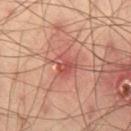Assessment:
Part of a total-body skin-imaging series; this lesion was reviewed on a skin check and was not flagged for biopsy.
Background:
A 15 mm close-up tile from a total-body photography series done for melanoma screening. Automated image analysis of the tile measured two-axis asymmetry of about 0.4. A male subject, aged around 40. On the right thigh. Captured under cross-polarized illumination. The recorded lesion diameter is about 2.5 mm.